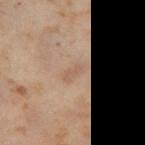Impression:
The lesion was photographed on a routine skin check and not biopsied; there is no pathology result.
Context:
On the right thigh. A close-up tile cropped from a whole-body skin photograph, about 15 mm across. The total-body-photography lesion software estimated an area of roughly 2.5 mm², a shape eccentricity near 0.9, and two-axis asymmetry of about 0.35. And it measured internal color variation of about 0 on a 0–10 scale and radial color variation of about 0. And it measured a classifier nevus-likeness of about 0/100 and a detector confidence of about 100 out of 100 that the crop contains a lesion. The subject is a female in their mid-50s. The recorded lesion diameter is about 2.5 mm.